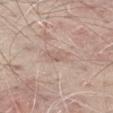Clinical impression:
This lesion was catalogued during total-body skin photography and was not selected for biopsy.
Image and clinical context:
A male subject in their mid-60s. On the leg. A close-up tile cropped from a whole-body skin photograph, about 15 mm across.